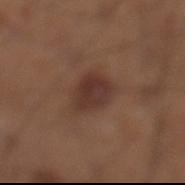The lesion was tiled from a total-body skin photograph and was not biopsied. Cropped from a total-body skin-imaging series; the visible field is about 15 mm. On the left lower leg. Approximately 4 mm at its widest. A male subject aged 58 to 62. An algorithmic analysis of the crop reported a lesion–skin lightness drop of about 9. And it measured a border-irregularity rating of about 2/10 and a peripheral color-asymmetry measure near 1. The analysis additionally found an automated nevus-likeness rating near 75 out of 100 and a lesion-detection confidence of about 100/100. Captured under white-light illumination.